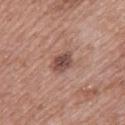Q: Was this lesion biopsied?
A: catalogued during a skin exam; not biopsied
Q: Automated lesion metrics?
A: a footprint of about 5 mm², an eccentricity of roughly 0.7, and two-axis asymmetry of about 0.2; an automated nevus-likeness rating near 40 out of 100 and lesion-presence confidence of about 100/100
Q: What is the imaging modality?
A: 15 mm crop, total-body photography
Q: How was the tile lit?
A: white-light
Q: Patient demographics?
A: female, in their 60s
Q: What is the anatomic site?
A: the upper back